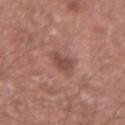<record>
<biopsy_status>not biopsied; imaged during a skin examination</biopsy_status>
<patient>
  <sex>male</sex>
  <age_approx>65</age_approx>
</patient>
<automated_metrics>
  <area_mm2_approx>5.0</area_mm2_approx>
  <eccentricity>0.4</eccentricity>
  <shape_asymmetry>0.3</shape_asymmetry>
  <cielab_L>48</cielab_L>
  <cielab_a>22</cielab_a>
  <cielab_b>24</cielab_b>
  <vs_skin_darker_L>9.0</vs_skin_darker_L>
  <vs_skin_contrast_norm>7.0</vs_skin_contrast_norm>
  <border_irregularity_0_10>3.0</border_irregularity_0_10>
  <color_variation_0_10>2.0</color_variation_0_10>
  <peripheral_color_asymmetry>1.0</peripheral_color_asymmetry>
  <nevus_likeness_0_100>10</nevus_likeness_0_100>
  <lesion_detection_confidence_0_100>100</lesion_detection_confidence_0_100>
</automated_metrics>
<lesion_size>
  <long_diameter_mm_approx>2.5</long_diameter_mm_approx>
</lesion_size>
<image>
  <source>total-body photography crop</source>
  <field_of_view_mm>15</field_of_view_mm>
</image>
<lighting>white-light</lighting>
<site>left forearm</site>
</record>